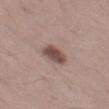biopsy_status: not biopsied; imaged during a skin examination
site: left thigh
lighting: white-light
patient:
  sex: male
  age_approx: 45
image:
  source: total-body photography crop
  field_of_view_mm: 15
automated_metrics:
  nevus_likeness_0_100: 80
  lesion_detection_confidence_0_100: 100